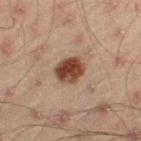Case summary:
• workup · no biopsy performed (imaged during a skin exam)
• tile lighting · cross-polarized
• size · about 3.5 mm
• patient · male, approximately 45 years of age
• image source · total-body-photography crop, ~15 mm field of view
• anatomic site · the right thigh